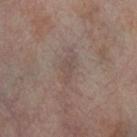{"biopsy_status": "not biopsied; imaged during a skin examination", "lesion_size": {"long_diameter_mm_approx": 3.5}, "patient": {"sex": "female", "age_approx": 55}, "site": "right thigh", "lighting": "cross-polarized", "image": {"source": "total-body photography crop", "field_of_view_mm": 15}}